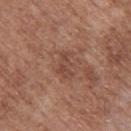Impression:
Captured during whole-body skin photography for melanoma surveillance; the lesion was not biopsied.
Context:
The lesion's longest dimension is about 3.5 mm. Located on the upper back. A roughly 15 mm field-of-view crop from a total-body skin photograph. Automated tile analysis of the lesion measured a mean CIELAB color near L≈46 a*≈21 b*≈27 and a lesion-to-skin contrast of about 5.5 (normalized; higher = more distinct). The tile uses white-light illumination. A male patient in their mid-70s.